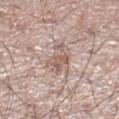Clinical impression:
Part of a total-body skin-imaging series; this lesion was reviewed on a skin check and was not flagged for biopsy.
Clinical summary:
Automated tile analysis of the lesion measured a footprint of about 7.5 mm², an eccentricity of roughly 0.75, and a shape-asymmetry score of about 0.35 (0 = symmetric). The software also gave border irregularity of about 5 on a 0–10 scale, a within-lesion color-variation index near 3/10, and radial color variation of about 1. This is a white-light tile. The lesion is on the right lower leg. A 15 mm close-up tile from a total-body photography series done for melanoma screening. A male subject, aged 58 to 62. About 4 mm across.